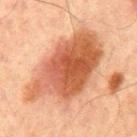Notes:
• biopsy status: catalogued during a skin exam; not biopsied
• image-analysis metrics: a mean CIELAB color near L≈47 a*≈23 b*≈31 and a normalized lesion–skin contrast near 9.5; a border-irregularity rating of about 4/10, internal color variation of about 6 on a 0–10 scale, and radial color variation of about 2
• anatomic site: the chest
• lesion diameter: about 10.5 mm
• lighting: cross-polarized
• patient: male, in their mid- to late 60s
• imaging modality: ~15 mm tile from a whole-body skin photo